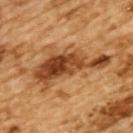location: the upper back; size: ~9 mm (longest diameter); illumination: cross-polarized; patient: male, in their mid-80s or older; image: ~15 mm crop, total-body skin-cancer survey.